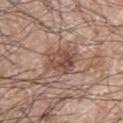Q: Where on the body is the lesion?
A: the left arm
Q: What are the patient's age and sex?
A: male, approximately 70 years of age
Q: What kind of image is this?
A: ~15 mm crop, total-body skin-cancer survey